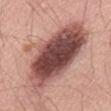biopsy status: imaged on a skin check; not biopsied | TBP lesion metrics: two-axis asymmetry of about 0.2; an average lesion color of about L≈49 a*≈23 b*≈23 (CIELAB), roughly 20 lightness units darker than nearby skin, and a normalized border contrast of about 13 | anatomic site: the abdomen | patient: male, roughly 60 years of age | acquisition: 15 mm crop, total-body photography | lighting: white-light | lesion size: ≈11.5 mm.